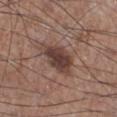follow-up: total-body-photography surveillance lesion; no biopsy
subject: male, about 65 years old
acquisition: total-body-photography crop, ~15 mm field of view
location: the right lower leg
lesion size: ~5.5 mm (longest diameter)
lighting: white-light illumination